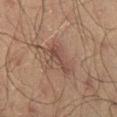  biopsy_status: not biopsied; imaged during a skin examination
  lighting: cross-polarized
  patient:
    sex: male
    age_approx: 70
  site: right thigh
  image:
    source: total-body photography crop
    field_of_view_mm: 15